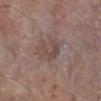  biopsy_status: not biopsied; imaged during a skin examination
  patient:
    sex: male
    age_approx: 85+
  site: leg
  lesion_size:
    long_diameter_mm_approx: 3.5
  lighting: white-light
  image:
    source: total-body photography crop
    field_of_view_mm: 15